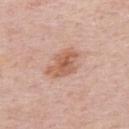<tbp_lesion>
<site>upper back</site>
<image>
  <source>total-body photography crop</source>
  <field_of_view_mm>15</field_of_view_mm>
</image>
<patient>
  <sex>male</sex>
  <age_approx>75</age_approx>
</patient>
<lesion_size>
  <long_diameter_mm_approx>5.0</long_diameter_mm_approx>
</lesion_size>
<automated_metrics>
  <area_mm2_approx>9.5</area_mm2_approx>
  <eccentricity>0.8</eccentricity>
  <shape_asymmetry>0.3</shape_asymmetry>
  <border_irregularity_0_10>3.0</border_irregularity_0_10>
  <color_variation_0_10>4.0</color_variation_0_10>
</automated_metrics>
</tbp_lesion>